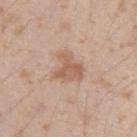The lesion was tiled from a total-body skin photograph and was not biopsied. A male subject aged around 25. The lesion-visualizer software estimated an eccentricity of roughly 0.45 and two-axis asymmetry of about 0.5. And it measured a mean CIELAB color near L≈59 a*≈19 b*≈30 and roughly 9 lightness units darker than nearby skin. And it measured a classifier nevus-likeness of about 15/100. Approximately 3.5 mm at its widest. On the left upper arm. A lesion tile, about 15 mm wide, cut from a 3D total-body photograph. Imaged with white-light lighting.